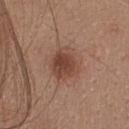follow-up: imaged on a skin check; not biopsied | anatomic site: the upper back | image: ~15 mm crop, total-body skin-cancer survey | patient: female, roughly 40 years of age | lighting: white-light.